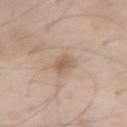Notes:
* workup · imaged on a skin check; not biopsied
* diameter · about 2.5 mm
* subject · male, approximately 70 years of age
* image source · 15 mm crop, total-body photography
* lighting · white-light
* anatomic site · the front of the torso
* automated lesion analysis · roughly 9 lightness units darker than nearby skin and a normalized lesion–skin contrast near 6.5